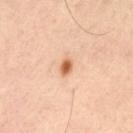follow-up = no biopsy performed (imaged during a skin exam) | patient = male, aged 63–67 | site = the left thigh | acquisition = ~15 mm crop, total-body skin-cancer survey | lesion size = ~2 mm (longest diameter) | illumination = cross-polarized | TBP lesion metrics = a border-irregularity rating of about 2/10, a within-lesion color-variation index near 2.5/10, and a peripheral color-asymmetry measure near 0.5.